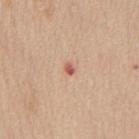The lesion was tiled from a total-body skin photograph and was not biopsied. The lesion is on the mid back. A female subject aged 53–57. A 15 mm close-up tile from a total-body photography series done for melanoma screening. Automated image analysis of the tile measured a mean CIELAB color near L≈58 a*≈30 b*≈28 and a normalized lesion–skin contrast near 8.5. The software also gave a within-lesion color-variation index near 0/10 and a peripheral color-asymmetry measure near 0. Approximately 1.5 mm at its widest. The tile uses white-light illumination.